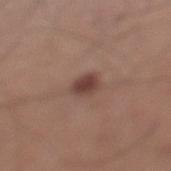notes = no biopsy performed (imaged during a skin exam) | acquisition = total-body-photography crop, ~15 mm field of view | patient = male, aged 53–57 | anatomic site = the left lower leg | lesion size = ~3 mm (longest diameter) | tile lighting = white-light.